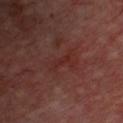• workup · imaged on a skin check; not biopsied